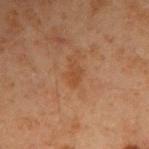Q: Is there a histopathology result?
A: catalogued during a skin exam; not biopsied
Q: What is the anatomic site?
A: the right upper arm
Q: What kind of image is this?
A: 15 mm crop, total-body photography
Q: What did automated image analysis measure?
A: an eccentricity of roughly 0.85 and a symmetry-axis asymmetry near 0.35; a mean CIELAB color near L≈35 a*≈18 b*≈29, about 5 CIELAB-L* units darker than the surrounding skin, and a lesion-to-skin contrast of about 5.5 (normalized; higher = more distinct); an automated nevus-likeness rating near 0 out of 100 and a detector confidence of about 100 out of 100 that the crop contains a lesion
Q: What is the lesion's diameter?
A: ≈2.5 mm
Q: Patient demographics?
A: male, aged 58 to 62